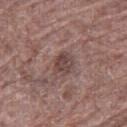Clinical impression: Imaged during a routine full-body skin examination; the lesion was not biopsied and no histopathology is available. Context: A male subject roughly 70 years of age. A lesion tile, about 15 mm wide, cut from a 3D total-body photograph. The lesion is on the right thigh.